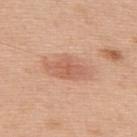Assessment: Captured during whole-body skin photography for melanoma surveillance; the lesion was not biopsied. Background: Cropped from a total-body skin-imaging series; the visible field is about 15 mm. A male subject approximately 70 years of age. The lesion is located on the back.